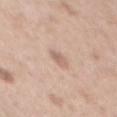Recorded during total-body skin imaging; not selected for excision or biopsy. The patient is a male aged around 55. A roughly 15 mm field-of-view crop from a total-body skin photograph. From the chest.